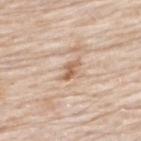biopsy status — no biopsy performed (imaged during a skin exam) | diameter — about 2.5 mm | subject — male, aged around 75 | body site — the upper back | imaging modality — ~15 mm tile from a whole-body skin photo | lighting — white-light illumination.